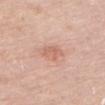lesion size — ~3 mm (longest diameter)
automated lesion analysis — an area of roughly 5 mm², an eccentricity of roughly 0.65, and a shape-asymmetry score of about 0.25 (0 = symmetric); a mean CIELAB color near L≈63 a*≈23 b*≈30, a lesion–skin lightness drop of about 8, and a lesion-to-skin contrast of about 5.5 (normalized; higher = more distinct); border irregularity of about 2.5 on a 0–10 scale, a color-variation rating of about 2/10, and peripheral color asymmetry of about 1
acquisition — ~15 mm crop, total-body skin-cancer survey
location — the upper back
subject — female, in their mid- to late 60s
tile lighting — white-light